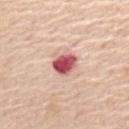This lesion was catalogued during total-body skin photography and was not selected for biopsy.
Imaged with white-light lighting.
A male subject aged 63 to 67.
From the upper back.
The recorded lesion diameter is about 3 mm.
A lesion tile, about 15 mm wide, cut from a 3D total-body photograph.
Automated image analysis of the tile measured border irregularity of about 2 on a 0–10 scale, a color-variation rating of about 4.5/10, and radial color variation of about 1.5.